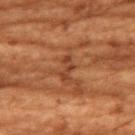Findings:
* workup: no biopsy performed (imaged during a skin exam)
* site: the chest
* diameter: about 2.5 mm
* tile lighting: cross-polarized illumination
* subject: female, aged approximately 80
* image: ~15 mm crop, total-body skin-cancer survey